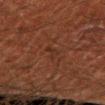Imaged during a routine full-body skin examination; the lesion was not biopsied and no histopathology is available. The lesion's longest dimension is about 2.5 mm. Cropped from a whole-body photographic skin survey; the tile spans about 15 mm. The lesion is on the right upper arm. A male patient, approximately 50 years of age.